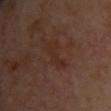{
  "lesion_size": {
    "long_diameter_mm_approx": 4.0
  },
  "lighting": "cross-polarized",
  "patient": {
    "sex": "female",
    "age_approx": 40
  },
  "automated_metrics": {
    "cielab_L": 28,
    "cielab_a": 19,
    "cielab_b": 24,
    "nevus_likeness_0_100": 0
  },
  "image": {
    "source": "total-body photography crop",
    "field_of_view_mm": 15
  },
  "site": "back"
}